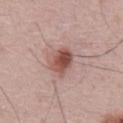{"site": "back", "patient": {"sex": "male", "age_approx": 65}, "image": {"source": "total-body photography crop", "field_of_view_mm": 15}}